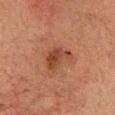The lesion was tiled from a total-body skin photograph and was not biopsied. Located on the head or neck. A roughly 15 mm field-of-view crop from a total-body skin photograph. Approximately 4.5 mm at its widest. The patient is a male aged around 65. Captured under cross-polarized illumination.